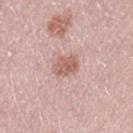Clinical impression: Part of a total-body skin-imaging series; this lesion was reviewed on a skin check and was not flagged for biopsy. Acquisition and patient details: The lesion is located on the left thigh. A male subject about 50 years old. This is a white-light tile. Longest diameter approximately 3 mm. A 15 mm crop from a total-body photograph taken for skin-cancer surveillance.